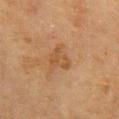Q: Was this lesion biopsied?
A: catalogued during a skin exam; not biopsied